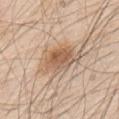| feature | finding |
|---|---|
| biopsy status | total-body-photography surveillance lesion; no biopsy |
| TBP lesion metrics | an outline eccentricity of about 0.85 (0 = round, 1 = elongated) and a shape-asymmetry score of about 0.25 (0 = symmetric); an average lesion color of about L≈61 a*≈17 b*≈31 (CIELAB) and a lesion–skin lightness drop of about 11; a classifier nevus-likeness of about 85/100 and a detector confidence of about 100 out of 100 that the crop contains a lesion |
| lesion diameter | ≈6.5 mm |
| location | the upper back |
| subject | male, aged 78–82 |
| lighting | white-light |
| imaging modality | 15 mm crop, total-body photography |